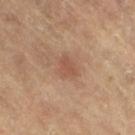Clinical impression: The lesion was photographed on a routine skin check and not biopsied; there is no pathology result. Clinical summary: The tile uses cross-polarized illumination. Approximately 2.5 mm at its widest. A female patient roughly 65 years of age. From the right thigh. Cropped from a whole-body photographic skin survey; the tile spans about 15 mm.